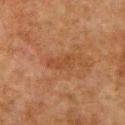Case summary:
• follow-up · catalogued during a skin exam; not biopsied
• image · 15 mm crop, total-body photography
• body site · the chest
• lighting · cross-polarized illumination
• subject · male, in their 80s